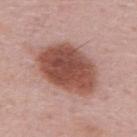biopsy status: imaged on a skin check; not biopsied | tile lighting: white-light illumination | TBP lesion metrics: a lesion area of about 29 mm², an eccentricity of roughly 0.75, and a shape-asymmetry score of about 0.1 (0 = symmetric); a lesion color around L≈50 a*≈24 b*≈26 in CIELAB; a nevus-likeness score of about 90/100 and lesion-presence confidence of about 100/100 | patient: male, aged 48 to 52 | acquisition: ~15 mm tile from a whole-body skin photo | body site: the upper back.